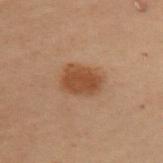Impression:
The lesion was tiled from a total-body skin photograph and was not biopsied.
Clinical summary:
A close-up tile cropped from a whole-body skin photograph, about 15 mm across. The patient is a female approximately 50 years of age. The tile uses cross-polarized illumination. Approximately 4 mm at its widest. On the left upper arm.